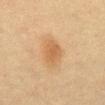Q: Is there a histopathology result?
A: total-body-photography surveillance lesion; no biopsy
Q: What are the patient's age and sex?
A: female, aged 53–57
Q: What kind of image is this?
A: 15 mm crop, total-body photography
Q: How large is the lesion?
A: ~3 mm (longest diameter)
Q: What is the anatomic site?
A: the abdomen
Q: Automated lesion metrics?
A: an area of roughly 6.5 mm², an eccentricity of roughly 0.55, and a shape-asymmetry score of about 0.15 (0 = symmetric); border irregularity of about 1.5 on a 0–10 scale, a color-variation rating of about 2/10, and radial color variation of about 0.5; a classifier nevus-likeness of about 85/100